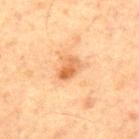Clinical impression: No biopsy was performed on this lesion — it was imaged during a full skin examination and was not determined to be concerning. Clinical summary: This is a cross-polarized tile. The lesion's longest dimension is about 3 mm. A lesion tile, about 15 mm wide, cut from a 3D total-body photograph. From the chest. A male subject, approximately 65 years of age.